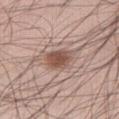Assessment: The lesion was photographed on a routine skin check and not biopsied; there is no pathology result. Background: The subject is a male approximately 45 years of age. The recorded lesion diameter is about 4 mm. From the right thigh. Automated image analysis of the tile measured an area of roughly 8 mm², a shape eccentricity near 0.75, and two-axis asymmetry of about 0.25. The software also gave a mean CIELAB color near L≈52 a*≈19 b*≈25 and a lesion–skin lightness drop of about 12. Cropped from a whole-body photographic skin survey; the tile spans about 15 mm.